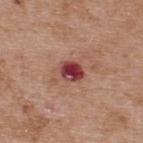follow-up=catalogued during a skin exam; not biopsied | site=the upper back | image-analysis metrics=a lesion color around L≈41 a*≈33 b*≈21 in CIELAB, about 17 CIELAB-L* units darker than the surrounding skin, and a normalized border contrast of about 13.5 | patient=male, approximately 55 years of age | image=~15 mm crop, total-body skin-cancer survey.